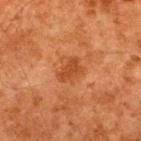follow-up: imaged on a skin check; not biopsied
patient: male, aged approximately 60
location: the back
illumination: cross-polarized illumination
image source: total-body-photography crop, ~15 mm field of view
TBP lesion metrics: a footprint of about 5 mm², an eccentricity of roughly 0.75, and a shape-asymmetry score of about 0.35 (0 = symmetric); a lesion color around L≈37 a*≈24 b*≈34 in CIELAB, a lesion–skin lightness drop of about 7, and a lesion-to-skin contrast of about 6.5 (normalized; higher = more distinct); a nevus-likeness score of about 5/100 and a lesion-detection confidence of about 100/100
lesion diameter: ≈3 mm